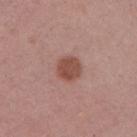biopsy_status: not biopsied; imaged during a skin examination
site: right upper arm
lighting: white-light
patient:
  sex: female
  age_approx: 50
lesion_size:
  long_diameter_mm_approx: 3.0
image:
  source: total-body photography crop
  field_of_view_mm: 15